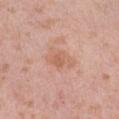Image and clinical context:
From the left thigh. A female subject in their 40s. A lesion tile, about 15 mm wide, cut from a 3D total-body photograph.